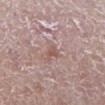This lesion was catalogued during total-body skin photography and was not selected for biopsy. The tile uses white-light illumination. A 15 mm close-up tile from a total-body photography series done for melanoma screening. A female subject, aged 63 to 67. The recorded lesion diameter is about 2.5 mm. Located on the right lower leg.